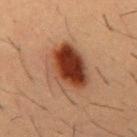{
  "biopsy_status": "not biopsied; imaged during a skin examination",
  "site": "chest",
  "image": {
    "source": "total-body photography crop",
    "field_of_view_mm": 15
  },
  "lesion_size": {
    "long_diameter_mm_approx": 6.5
  },
  "patient": {
    "sex": "male",
    "age_approx": 55
  }
}